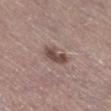Case summary:
- follow-up · total-body-photography surveillance lesion; no biopsy
- illumination · white-light illumination
- subject · male, in their mid-60s
- size · about 4 mm
- body site · the right lower leg
- imaging modality · 15 mm crop, total-body photography